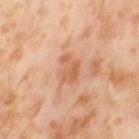Clinical impression: The lesion was photographed on a routine skin check and not biopsied; there is no pathology result. Image and clinical context: The tile uses cross-polarized illumination. The lesion's longest dimension is about 4 mm. The subject is a female aged around 55. The lesion is located on the left thigh. An algorithmic analysis of the crop reported roughly 9 lightness units darker than nearby skin and a lesion-to-skin contrast of about 6 (normalized; higher = more distinct). The software also gave a nevus-likeness score of about 0/100 and a detector confidence of about 100 out of 100 that the crop contains a lesion. A 15 mm close-up extracted from a 3D total-body photography capture.